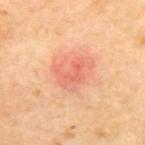Q: Is there a histopathology result?
A: imaged on a skin check; not biopsied
Q: Patient demographics?
A: male, in their mid- to late 60s
Q: How was this image acquired?
A: total-body-photography crop, ~15 mm field of view
Q: Illumination type?
A: cross-polarized
Q: What is the anatomic site?
A: the upper back
Q: Lesion size?
A: about 4.5 mm